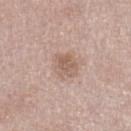{
  "biopsy_status": "not biopsied; imaged during a skin examination",
  "patient": {
    "sex": "male",
    "age_approx": 70
  },
  "site": "left lower leg",
  "image": {
    "source": "total-body photography crop",
    "field_of_view_mm": 15
  }
}